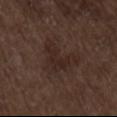{"biopsy_status": "not biopsied; imaged during a skin examination", "image": {"source": "total-body photography crop", "field_of_view_mm": 15}, "automated_metrics": {"area_mm2_approx": 12.0, "eccentricity": 0.7, "shape_asymmetry": 0.6, "cielab_L": 25, "cielab_a": 15, "cielab_b": 19, "vs_skin_darker_L": 5.0, "vs_skin_contrast_norm": 6.0, "color_variation_0_10": 2.5, "peripheral_color_asymmetry": 1.0, "nevus_likeness_0_100": 5, "lesion_detection_confidence_0_100": 100}, "patient": {"sex": "male", "age_approx": 70}, "lesion_size": {"long_diameter_mm_approx": 5.5}, "lighting": "white-light", "site": "mid back"}